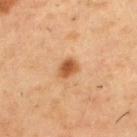No biopsy was performed on this lesion — it was imaged during a full skin examination and was not determined to be concerning. Automated tile analysis of the lesion measured an area of roughly 4 mm², a shape eccentricity near 0.65, and two-axis asymmetry of about 0.2. And it measured a lesion-detection confidence of about 100/100. The lesion is located on the upper back. A 15 mm close-up tile from a total-body photography series done for melanoma screening. A male patient, aged around 55. Approximately 2.5 mm at its widest.